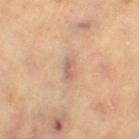Impression: This lesion was catalogued during total-body skin photography and was not selected for biopsy. Image and clinical context: Cropped from a whole-body photographic skin survey; the tile spans about 15 mm. The lesion-visualizer software estimated roughly 9 lightness units darker than nearby skin and a lesion-to-skin contrast of about 6.5 (normalized; higher = more distinct). It also reported a border-irregularity rating of about 2.5/10 and a peripheral color-asymmetry measure near 0.5. The analysis additionally found a nevus-likeness score of about 0/100 and a lesion-detection confidence of about 95/100. About 3 mm across. The lesion is on the leg. A female patient aged 68–72.